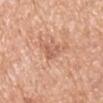follow-up=imaged on a skin check; not biopsied
patient=male, in their mid- to late 60s
site=the arm
image source=total-body-photography crop, ~15 mm field of view